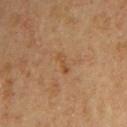Imaged during a routine full-body skin examination; the lesion was not biopsied and no histopathology is available. Cropped from a whole-body photographic skin survey; the tile spans about 15 mm. Captured under cross-polarized illumination. Measured at roughly 3 mm in maximum diameter. A male patient about 60 years old. An algorithmic analysis of the crop reported an area of roughly 3 mm², an eccentricity of roughly 0.9, and a symmetry-axis asymmetry near 0.35. It also reported about 5 CIELAB-L* units darker than the surrounding skin. And it measured a border-irregularity index near 4/10, internal color variation of about 0 on a 0–10 scale, and a peripheral color-asymmetry measure near 0. It also reported an automated nevus-likeness rating near 0 out of 100 and a detector confidence of about 100 out of 100 that the crop contains a lesion.